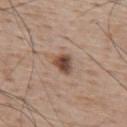Clinical impression: No biopsy was performed on this lesion — it was imaged during a full skin examination and was not determined to be concerning. Background: The subject is a male in their mid-60s. Longest diameter approximately 3 mm. On the upper back. Captured under white-light illumination. The lesion-visualizer software estimated an area of roughly 5 mm², an outline eccentricity of about 0.6 (0 = round, 1 = elongated), and two-axis asymmetry of about 0.25. It also reported an average lesion color of about L≈47 a*≈18 b*≈26 (CIELAB), about 14 CIELAB-L* units darker than the surrounding skin, and a normalized lesion–skin contrast near 10. A 15 mm close-up tile from a total-body photography series done for melanoma screening.